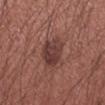No biopsy was performed on this lesion — it was imaged during a full skin examination and was not determined to be concerning. The patient is a male approximately 35 years of age. The lesion is located on the left forearm. A roughly 15 mm field-of-view crop from a total-body skin photograph.